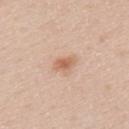The patient is a male approximately 40 years of age.
This image is a 15 mm lesion crop taken from a total-body photograph.
Approximately 2.5 mm at its widest.
The lesion is on the upper back.
Automated tile analysis of the lesion measured an area of roughly 4 mm² and a symmetry-axis asymmetry near 0.15. And it measured a border-irregularity index near 1.5/10, a color-variation rating of about 3/10, and peripheral color asymmetry of about 1.